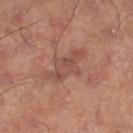Q: Is there a histopathology result?
A: no biopsy performed (imaged during a skin exam)
Q: How was this image acquired?
A: ~15 mm tile from a whole-body skin photo
Q: Where on the body is the lesion?
A: the leg
Q: Illumination type?
A: cross-polarized
Q: What is the lesion's diameter?
A: about 5.5 mm